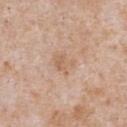Captured during whole-body skin photography for melanoma surveillance; the lesion was not biopsied. A male subject in their mid- to late 60s. From the chest. A 15 mm close-up tile from a total-body photography series done for melanoma screening. The recorded lesion diameter is about 3 mm. Automated tile analysis of the lesion measured an average lesion color of about L≈62 a*≈18 b*≈31 (CIELAB), a lesion–skin lightness drop of about 7, and a normalized border contrast of about 5. It also reported a classifier nevus-likeness of about 0/100 and lesion-presence confidence of about 100/100.